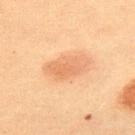Q: Was this lesion biopsied?
A: total-body-photography surveillance lesion; no biopsy
Q: What is the lesion's diameter?
A: ≈5.5 mm
Q: How was this image acquired?
A: total-body-photography crop, ~15 mm field of view
Q: Where on the body is the lesion?
A: the back
Q: What are the patient's age and sex?
A: male, aged 58–62
Q: Illumination type?
A: cross-polarized illumination